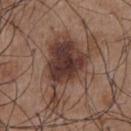Impression: This lesion was catalogued during total-body skin photography and was not selected for biopsy. Context: The patient is a male roughly 55 years of age. About 10.5 mm across. The lesion is located on the abdomen. Captured under white-light illumination. A 15 mm close-up extracted from a 3D total-body photography capture.